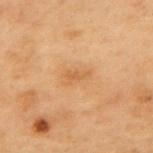Imaged during a routine full-body skin examination; the lesion was not biopsied and no histopathology is available.
A male subject, in their mid- to late 50s.
The lesion is located on the upper back.
A 15 mm crop from a total-body photograph taken for skin-cancer surveillance.